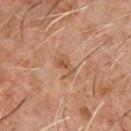Impression: This lesion was catalogued during total-body skin photography and was not selected for biopsy. Image and clinical context: About 3 mm across. A region of skin cropped from a whole-body photographic capture, roughly 15 mm wide. Captured under cross-polarized illumination. A male subject, roughly 60 years of age. On the chest.